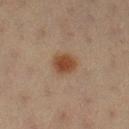No biopsy was performed on this lesion — it was imaged during a full skin examination and was not determined to be concerning. On the left lower leg. The total-body-photography lesion software estimated an eccentricity of roughly 0.6 and two-axis asymmetry of about 0.2. And it measured roughly 10 lightness units darker than nearby skin. The analysis additionally found a border-irregularity index near 2/10, a color-variation rating of about 2.5/10, and a peripheral color-asymmetry measure near 0.5. A 15 mm close-up extracted from a 3D total-body photography capture. The patient is a female in their 20s. Approximately 3 mm at its widest. The tile uses cross-polarized illumination.